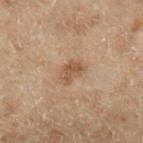On the left lower leg. Approximately 3 mm at its widest. A 15 mm crop from a total-body photograph taken for skin-cancer surveillance. An algorithmic analysis of the crop reported an outline eccentricity of about 0.75 (0 = round, 1 = elongated) and a shape-asymmetry score of about 0.3 (0 = symmetric). It also reported a lesion color around L≈39 a*≈14 b*≈25 in CIELAB, a lesion–skin lightness drop of about 7, and a lesion-to-skin contrast of about 6.5 (normalized; higher = more distinct). The software also gave a border-irregularity index near 3/10, internal color variation of about 2 on a 0–10 scale, and peripheral color asymmetry of about 1. A male subject about 70 years old. This is a cross-polarized tile.